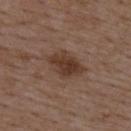workup: catalogued during a skin exam; not biopsied | acquisition: total-body-photography crop, ~15 mm field of view | lesion diameter: about 4.5 mm | automated metrics: an average lesion color of about L≈37 a*≈18 b*≈25 (CIELAB), roughly 10 lightness units darker than nearby skin, and a normalized border contrast of about 9; a border-irregularity rating of about 2/10; a nevus-likeness score of about 80/100 | body site: the back | subject: male, in their 50s.